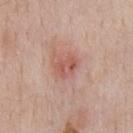workup: imaged on a skin check; not biopsied
location: the chest
acquisition: ~15 mm crop, total-body skin-cancer survey
patient: male, aged around 60